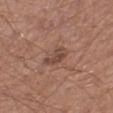notes = total-body-photography surveillance lesion; no biopsy
automated lesion analysis = border irregularity of about 3.5 on a 0–10 scale, internal color variation of about 3.5 on a 0–10 scale, and peripheral color asymmetry of about 1.5; a classifier nevus-likeness of about 20/100 and a detector confidence of about 100 out of 100 that the crop contains a lesion
location = the leg
image source = ~15 mm crop, total-body skin-cancer survey
subject = male, about 65 years old
tile lighting = white-light illumination
size = ~3 mm (longest diameter)